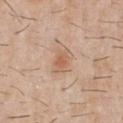Q: Was this lesion biopsied?
A: no biopsy performed (imaged during a skin exam)